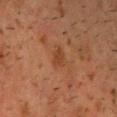notes=imaged on a skin check; not biopsied | acquisition=total-body-photography crop, ~15 mm field of view | site=the head or neck | patient=male, aged approximately 50 | lesion size=≈2.5 mm.